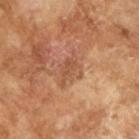No biopsy was performed on this lesion — it was imaged during a full skin examination and was not determined to be concerning. The patient is a male about 65 years old. A lesion tile, about 15 mm wide, cut from a 3D total-body photograph. Imaged with cross-polarized lighting. About 4 mm across.